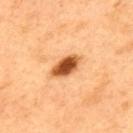Case summary:
- workup · catalogued during a skin exam; not biopsied
- body site · the upper back
- automated metrics · a lesion area of about 7 mm² and an outline eccentricity of about 0.85 (0 = round, 1 = elongated); an average lesion color of about L≈56 a*≈29 b*≈45 (CIELAB) and a lesion–skin lightness drop of about 21; a border-irregularity rating of about 1.5/10, a color-variation rating of about 5/10, and a peripheral color-asymmetry measure near 1
- lighting · cross-polarized illumination
- lesion diameter · ≈4 mm
- image · 15 mm crop, total-body photography
- subject · male, about 50 years old